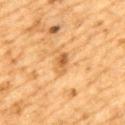The lesion was tiled from a total-body skin photograph and was not biopsied.
About 3 mm across.
The lesion is located on the mid back.
This image is a 15 mm lesion crop taken from a total-body photograph.
A male subject, aged 83 or older.
The total-body-photography lesion software estimated a mean CIELAB color near L≈53 a*≈21 b*≈41 and a lesion–skin lightness drop of about 10. The analysis additionally found a nevus-likeness score of about 45/100 and a lesion-detection confidence of about 100/100.
This is a cross-polarized tile.